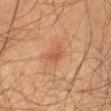No biopsy was performed on this lesion — it was imaged during a full skin examination and was not determined to be concerning. The total-body-photography lesion software estimated a border-irregularity index near 4/10, internal color variation of about 1.5 on a 0–10 scale, and radial color variation of about 0.5. The analysis additionally found an automated nevus-likeness rating near 10 out of 100 and a lesion-detection confidence of about 100/100. A close-up tile cropped from a whole-body skin photograph, about 15 mm across. Approximately 2.5 mm at its widest. On the front of the torso. A male subject in their 60s. The tile uses cross-polarized illumination.